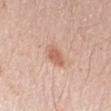Impression:
The lesion was tiled from a total-body skin photograph and was not biopsied.
Background:
A female subject, in their 40s. Located on the left forearm. The tile uses white-light illumination. About 3 mm across. A lesion tile, about 15 mm wide, cut from a 3D total-body photograph.